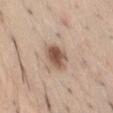follow-up — total-body-photography surveillance lesion; no biopsy | location — the abdomen | lighting — white-light illumination | imaging modality — ~15 mm tile from a whole-body skin photo | diameter — ≈4 mm | subject — male, in their mid- to late 30s.